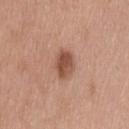No biopsy was performed on this lesion — it was imaged during a full skin examination and was not determined to be concerning.
A region of skin cropped from a whole-body photographic capture, roughly 15 mm wide.
Longest diameter approximately 3.5 mm.
A male patient, aged 43 to 47.
The lesion is located on the upper back.
The tile uses white-light illumination.